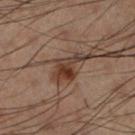follow-up = no biopsy performed (imaged during a skin exam) | image source = ~15 mm tile from a whole-body skin photo | lesion size = ~5 mm (longest diameter) | location = the left lower leg | illumination = cross-polarized illumination | subject = male, approximately 50 years of age.